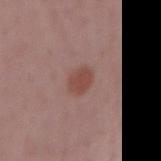Impression:
Captured during whole-body skin photography for melanoma surveillance; the lesion was not biopsied.
Context:
The lesion is on the right forearm. Imaged with white-light lighting. Longest diameter approximately 2.5 mm. A roughly 15 mm field-of-view crop from a total-body skin photograph. A male subject aged 68 to 72.